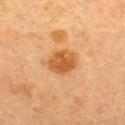workup: catalogued during a skin exam; not biopsied | lighting: cross-polarized | patient: female, about 60 years old | acquisition: ~15 mm tile from a whole-body skin photo | site: the upper back.